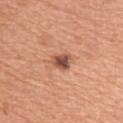Case summary:
– biopsy status — no biopsy performed (imaged during a skin exam)
– acquisition — ~15 mm crop, total-body skin-cancer survey
– patient — female, aged approximately 60
– location — the left upper arm
– lesion size — ≈2.5 mm
– tile lighting — white-light
– TBP lesion metrics — a footprint of about 4 mm², an outline eccentricity of about 0.55 (0 = round, 1 = elongated), and two-axis asymmetry of about 0.35; a color-variation rating of about 4.5/10 and radial color variation of about 1.5; an automated nevus-likeness rating near 20 out of 100 and lesion-presence confidence of about 100/100